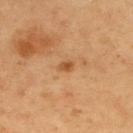Clinical impression: No biopsy was performed on this lesion — it was imaged during a full skin examination and was not determined to be concerning. Acquisition and patient details: On the back. The subject is a female aged 58 to 62. The lesion's longest dimension is about 2 mm. A 15 mm crop from a total-body photograph taken for skin-cancer surveillance. Captured under cross-polarized illumination. Automated image analysis of the tile measured a lesion area of about 2 mm². The software also gave a lesion–skin lightness drop of about 11. The analysis additionally found border irregularity of about 2 on a 0–10 scale, a color-variation rating of about 0.5/10, and radial color variation of about 0.